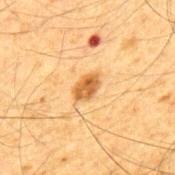{"biopsy_status": "not biopsied; imaged during a skin examination", "site": "upper back", "patient": {"sex": "male", "age_approx": 65}, "automated_metrics": {"area_mm2_approx": 4.5, "eccentricity": 0.85, "shape_asymmetry": 0.2}, "image": {"source": "total-body photography crop", "field_of_view_mm": 15}}